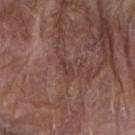No biopsy was performed on this lesion — it was imaged during a full skin examination and was not determined to be concerning.
Located on the right forearm.
The tile uses white-light illumination.
The patient is a male in their mid- to late 80s or older.
A roughly 15 mm field-of-view crop from a total-body skin photograph.
Automated tile analysis of the lesion measured a footprint of about 2.5 mm² and two-axis asymmetry of about 0.35. It also reported an average lesion color of about L≈39 a*≈20 b*≈22 (CIELAB), roughly 6 lightness units darker than nearby skin, and a lesion-to-skin contrast of about 5 (normalized; higher = more distinct). It also reported a nevus-likeness score of about 0/100 and a detector confidence of about 70 out of 100 that the crop contains a lesion.